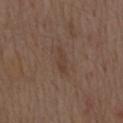Impression: This lesion was catalogued during total-body skin photography and was not selected for biopsy. Image and clinical context: A male patient aged 68 to 72. Captured under white-light illumination. From the mid back. A region of skin cropped from a whole-body photographic capture, roughly 15 mm wide. About 3 mm across.